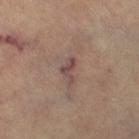follow-up: catalogued during a skin exam; not biopsied
illumination: cross-polarized illumination
site: the right lower leg
image: total-body-photography crop, ~15 mm field of view
subject: female, roughly 65 years of age